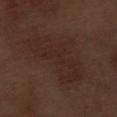Part of a total-body skin-imaging series; this lesion was reviewed on a skin check and was not flagged for biopsy. Measured at roughly 9 mm in maximum diameter. This is a white-light tile. The lesion is on the left thigh. The subject is a male aged 68 to 72. A close-up tile cropped from a whole-body skin photograph, about 15 mm across.